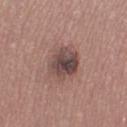Findings:
– biopsy status: no biopsy performed (imaged during a skin exam)
– automated lesion analysis: a lesion area of about 13 mm² and a shape eccentricity near 0.7; an automated nevus-likeness rating near 20 out of 100 and a lesion-detection confidence of about 100/100
– lighting: white-light
– lesion diameter: ~5 mm (longest diameter)
– subject: female, about 35 years old
– site: the right thigh
– image source: ~15 mm tile from a whole-body skin photo